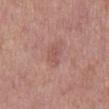Recorded during total-body skin imaging; not selected for excision or biopsy.